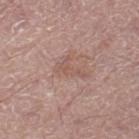This lesion was catalogued during total-body skin photography and was not selected for biopsy. Cropped from a total-body skin-imaging series; the visible field is about 15 mm. The recorded lesion diameter is about 4 mm. This is a white-light tile. The lesion is on the right lower leg. The subject is a male aged 58 to 62.